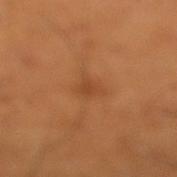Part of a total-body skin-imaging series; this lesion was reviewed on a skin check and was not flagged for biopsy.
The patient is a male approximately 55 years of age.
Automated tile analysis of the lesion measured a lesion area of about 5 mm², a shape eccentricity near 0.4, and two-axis asymmetry of about 0.35. The analysis additionally found a mean CIELAB color near L≈44 a*≈23 b*≈37 and about 6 CIELAB-L* units darker than the surrounding skin. And it measured a border-irregularity rating of about 3.5/10 and a peripheral color-asymmetry measure near 0.5.
The lesion is located on the right lower leg.
A roughly 15 mm field-of-view crop from a total-body skin photograph.
Captured under cross-polarized illumination.
Longest diameter approximately 3 mm.